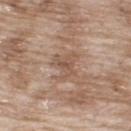Findings:
– notes · imaged on a skin check; not biopsied
– diameter · ≈4 mm
– lighting · white-light
– anatomic site · the upper back
– imaging modality · total-body-photography crop, ~15 mm field of view
– patient · male, approximately 65 years of age